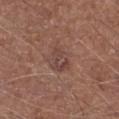Impression: Part of a total-body skin-imaging series; this lesion was reviewed on a skin check and was not flagged for biopsy. Image and clinical context: The total-body-photography lesion software estimated an area of roughly 7 mm², a shape eccentricity near 0.65, and a shape-asymmetry score of about 0.3 (0 = symmetric). It also reported a mean CIELAB color near L≈43 a*≈19 b*≈22, roughly 7 lightness units darker than nearby skin, and a normalized lesion–skin contrast near 5.5. It also reported a border-irregularity index near 3/10. The analysis additionally found a detector confidence of about 100 out of 100 that the crop contains a lesion. A region of skin cropped from a whole-body photographic capture, roughly 15 mm wide. The lesion's longest dimension is about 3.5 mm. On the leg. A male patient, aged approximately 75.